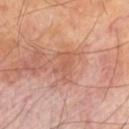No biopsy was performed on this lesion — it was imaged during a full skin examination and was not determined to be concerning. This is a cross-polarized tile. A 15 mm close-up tile from a total-body photography series done for melanoma screening. On the left thigh. A male patient approximately 70 years of age. The lesion's longest dimension is about 3.5 mm.